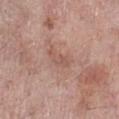biopsy_status: not biopsied; imaged during a skin examination
automated_metrics:
  area_mm2_approx: 5.0
  eccentricity: 0.85
  shape_asymmetry: 0.45
  cielab_L: 55
  cielab_a: 20
  cielab_b: 26
  border_irregularity_0_10: 4.5
  color_variation_0_10: 2.0
  peripheral_color_asymmetry: 0.5
  nevus_likeness_0_100: 0
  lesion_detection_confidence_0_100: 100
lesion_size:
  long_diameter_mm_approx: 3.5
site: right lower leg
lighting: white-light
image:
  source: total-body photography crop
  field_of_view_mm: 15
patient:
  sex: female
  age_approx: 70